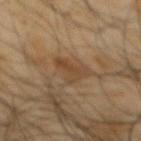<case>
<image>
  <source>total-body photography crop</source>
  <field_of_view_mm>15</field_of_view_mm>
</image>
<patient>
  <sex>male</sex>
  <age_approx>65</age_approx>
</patient>
<lesion_size>
  <long_diameter_mm_approx>3.5</long_diameter_mm_approx>
</lesion_size>
<lighting>cross-polarized</lighting>
<site>mid back</site>
</case>